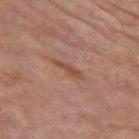Captured during whole-body skin photography for melanoma surveillance; the lesion was not biopsied.
The tile uses cross-polarized illumination.
Measured at roughly 3 mm in maximum diameter.
The lesion-visualizer software estimated an eccentricity of roughly 0.95. And it measured a lesion color around L≈42 a*≈21 b*≈27 in CIELAB, a lesion–skin lightness drop of about 8, and a normalized border contrast of about 7.
A 15 mm close-up tile from a total-body photography series done for melanoma screening.
On the right leg.
The subject is a female aged 78 to 82.